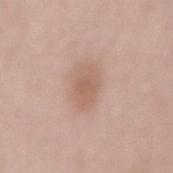Case summary:
– notes · catalogued during a skin exam; not biopsied
– illumination · white-light
– lesion size · ~4.5 mm (longest diameter)
– patient · female, about 70 years old
– anatomic site · the lower back
– acquisition · ~15 mm crop, total-body skin-cancer survey